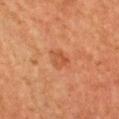image source: ~15 mm tile from a whole-body skin photo | anatomic site: the chest | patient: female, about 60 years old | illumination: cross-polarized | diameter: ~2.5 mm (longest diameter) | TBP lesion metrics: a footprint of about 4.5 mm² and an outline eccentricity of about 0.55 (0 = round, 1 = elongated); a mean CIELAB color near L≈47 a*≈25 b*≈35, roughly 7 lightness units darker than nearby skin, and a lesion-to-skin contrast of about 5.5 (normalized; higher = more distinct); a border-irregularity rating of about 3.5/10 and a color-variation rating of about 2/10; a detector confidence of about 100 out of 100 that the crop contains a lesion.